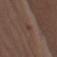notes: no biopsy performed (imaged during a skin exam) | automated metrics: an area of roughly 2.5 mm², an outline eccentricity of about 0.95 (0 = round, 1 = elongated), and two-axis asymmetry of about 0.4; a mean CIELAB color near L≈38 a*≈18 b*≈22, a lesion–skin lightness drop of about 5, and a normalized border contrast of about 5 | illumination: white-light illumination | subject: male, approximately 75 years of age | lesion size: ~3.5 mm (longest diameter) | location: the right upper arm | imaging modality: ~15 mm crop, total-body skin-cancer survey.